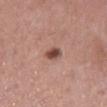Acquisition and patient details: A 15 mm close-up extracted from a 3D total-body photography capture. From the left lower leg. The subject is a female about 60 years old. This is a white-light tile. An algorithmic analysis of the crop reported a lesion color around L≈47 a*≈23 b*≈25 in CIELAB and roughly 14 lightness units darker than nearby skin.